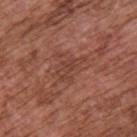This lesion was catalogued during total-body skin photography and was not selected for biopsy.
The recorded lesion diameter is about 3.5 mm.
On the upper back.
A roughly 15 mm field-of-view crop from a total-body skin photograph.
A male patient in their mid- to late 70s.